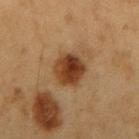The lesion was photographed on a routine skin check and not biopsied; there is no pathology result. The tile uses cross-polarized illumination. Approximately 4 mm at its widest. A 15 mm close-up tile from a total-body photography series done for melanoma screening. The subject is a male in their 60s. The lesion is on the right upper arm.